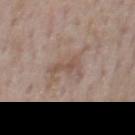<record>
<patient>
  <sex>male</sex>
  <age_approx>75</age_approx>
</patient>
<site>front of the torso</site>
<image>
  <source>total-body photography crop</source>
  <field_of_view_mm>15</field_of_view_mm>
</image>
<lighting>white-light</lighting>
<lesion_size>
  <long_diameter_mm_approx>4.0</long_diameter_mm_approx>
</lesion_size>
<automated_metrics>
  <border_irregularity_0_10>7.5</border_irregularity_0_10>
  <color_variation_0_10>1.0</color_variation_0_10>
  <peripheral_color_asymmetry>0.0</peripheral_color_asymmetry>
</automated_metrics>
</record>